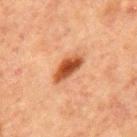Recorded during total-body skin imaging; not selected for excision or biopsy. This image is a 15 mm lesion crop taken from a total-body photograph. On the chest. A male subject approximately 65 years of age. The lesion's longest dimension is about 4 mm. Imaged with cross-polarized lighting.